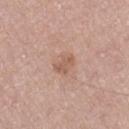| field | value |
|---|---|
| workup | total-body-photography surveillance lesion; no biopsy |
| diameter | ~2.5 mm (longest diameter) |
| subject | female, in their 50s |
| TBP lesion metrics | a mean CIELAB color near L≈57 a*≈21 b*≈28, roughly 8 lightness units darker than nearby skin, and a lesion-to-skin contrast of about 6 (normalized; higher = more distinct); border irregularity of about 3 on a 0–10 scale, a color-variation rating of about 1.5/10, and radial color variation of about 0.5; a classifier nevus-likeness of about 5/100 and lesion-presence confidence of about 100/100 |
| illumination | white-light |
| location | the left forearm |
| acquisition | total-body-photography crop, ~15 mm field of view |